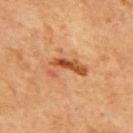Q: How was this image acquired?
A: total-body-photography crop, ~15 mm field of view
Q: Lesion location?
A: the back
Q: Who is the patient?
A: male, in their mid-60s
Q: Illumination type?
A: cross-polarized illumination